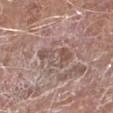Clinical impression:
Recorded during total-body skin imaging; not selected for excision or biopsy.
Clinical summary:
An algorithmic analysis of the crop reported a shape-asymmetry score of about 0.4 (0 = symmetric). The analysis additionally found an average lesion color of about L≈52 a*≈17 b*≈22 (CIELAB), about 8 CIELAB-L* units darker than the surrounding skin, and a normalized lesion–skin contrast near 6. And it measured border irregularity of about 5 on a 0–10 scale, a color-variation rating of about 5/10, and radial color variation of about 1. It also reported a classifier nevus-likeness of about 0/100 and a lesion-detection confidence of about 65/100. On the leg. A male subject, approximately 80 years of age. A close-up tile cropped from a whole-body skin photograph, about 15 mm across.